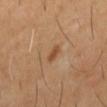notes: imaged on a skin check; not biopsied
subject: male, about 60 years old
image: total-body-photography crop, ~15 mm field of view
anatomic site: the left thigh